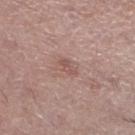follow-up: catalogued during a skin exam; not biopsied | illumination: white-light | image source: ~15 mm crop, total-body skin-cancer survey | subject: female, aged around 65 | location: the left lower leg | size: about 2.5 mm.